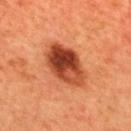Imaged during a routine full-body skin examination; the lesion was not biopsied and no histopathology is available. A female patient, aged around 55. The lesion is located on the upper back. The tile uses cross-polarized illumination. The lesion's longest dimension is about 6 mm. A region of skin cropped from a whole-body photographic capture, roughly 15 mm wide.